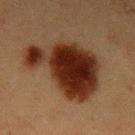biopsy_status: not biopsied; imaged during a skin examination
patient:
  sex: male
  age_approx: 40
lighting: cross-polarized
lesion_size:
  long_diameter_mm_approx: 8.5
site: left upper arm
image:
  source: total-body photography crop
  field_of_view_mm: 15